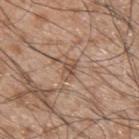Recorded during total-body skin imaging; not selected for excision or biopsy. On the back. The lesion-visualizer software estimated a lesion-to-skin contrast of about 6 (normalized; higher = more distinct). The software also gave an automated nevus-likeness rating near 0 out of 100 and a detector confidence of about 55 out of 100 that the crop contains a lesion. A roughly 15 mm field-of-view crop from a total-body skin photograph. A male subject, aged approximately 45.